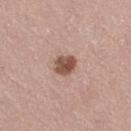Findings:
– workup · total-body-photography surveillance lesion; no biopsy
– image · ~15 mm tile from a whole-body skin photo
– location · the right thigh
– diameter · ~3 mm (longest diameter)
– subject · female, aged 48–52